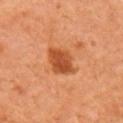The lesion was tiled from a total-body skin photograph and was not biopsied. A female patient, in their mid- to late 50s. A 15 mm close-up tile from a total-body photography series done for melanoma screening. An algorithmic analysis of the crop reported an area of roughly 10 mm², an outline eccentricity of about 0.65 (0 = round, 1 = elongated), and two-axis asymmetry of about 0.25. And it measured a color-variation rating of about 3.5/10 and radial color variation of about 1. And it measured an automated nevus-likeness rating near 95 out of 100. From the right upper arm. About 4 mm across.